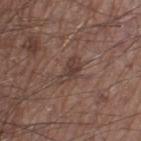notes: total-body-photography surveillance lesion; no biopsy.